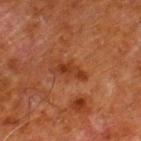Longest diameter approximately 3.5 mm.
A male subject, aged around 80.
The lesion is on the right lower leg.
A 15 mm crop from a total-body photograph taken for skin-cancer surveillance.